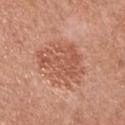<lesion>
<biopsy_status>not biopsied; imaged during a skin examination</biopsy_status>
<automated_metrics>
  <border_irregularity_0_10>6.0</border_irregularity_0_10>
  <color_variation_0_10>3.5</color_variation_0_10>
  <peripheral_color_asymmetry>1.5</peripheral_color_asymmetry>
  <nevus_likeness_0_100>5</nevus_likeness_0_100>
  <lesion_detection_confidence_0_100>100</lesion_detection_confidence_0_100>
</automated_metrics>
<lesion_size>
  <long_diameter_mm_approx>6.5</long_diameter_mm_approx>
</lesion_size>
<lighting>white-light</lighting>
<image>
  <source>total-body photography crop</source>
  <field_of_view_mm>15</field_of_view_mm>
</image>
<patient>
  <sex>male</sex>
  <age_approx>55</age_approx>
</patient>
<site>chest</site>
</lesion>